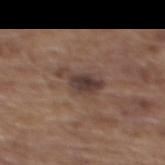Part of a total-body skin-imaging series; this lesion was reviewed on a skin check and was not flagged for biopsy.
A female patient, aged approximately 65.
Approximately 4.5 mm at its widest.
From the back.
This is a white-light tile.
A close-up tile cropped from a whole-body skin photograph, about 15 mm across.